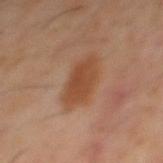This lesion was catalogued during total-body skin photography and was not selected for biopsy.